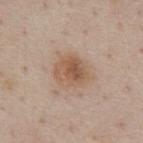Clinical impression: Recorded during total-body skin imaging; not selected for excision or biopsy. Acquisition and patient details: The lesion's longest dimension is about 4.5 mm. The subject is a male aged 58 to 62. From the front of the torso. Captured under white-light illumination. Cropped from a total-body skin-imaging series; the visible field is about 15 mm.